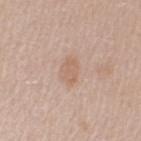Notes:
– size — about 3 mm
– image — 15 mm crop, total-body photography
– body site — the left upper arm
– lighting — white-light
– subject — female, aged 38 to 42
– image-analysis metrics — a border-irregularity index near 2.5/10, internal color variation of about 1.5 on a 0–10 scale, and a peripheral color-asymmetry measure near 0.5